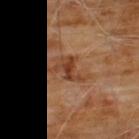Assessment: The lesion was photographed on a routine skin check and not biopsied; there is no pathology result. Context: An algorithmic analysis of the crop reported a mean CIELAB color near L≈36 a*≈23 b*≈32, roughly 11 lightness units darker than nearby skin, and a lesion-to-skin contrast of about 9 (normalized; higher = more distinct). And it measured a border-irregularity index near 5/10 and peripheral color asymmetry of about 0. Captured under cross-polarized illumination. The patient is a male in their 60s. The lesion is on the chest. A 15 mm close-up tile from a total-body photography series done for melanoma screening.